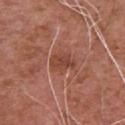Notes:
* workup: catalogued during a skin exam; not biopsied
* image source: ~15 mm tile from a whole-body skin photo
* illumination: white-light
* automated metrics: a mean CIELAB color near L≈44 a*≈25 b*≈29, a lesion–skin lightness drop of about 8, and a normalized border contrast of about 6.5; border irregularity of about 2.5 on a 0–10 scale; a nevus-likeness score of about 5/100 and a detector confidence of about 100 out of 100 that the crop contains a lesion
* lesion size: ≈3 mm
* patient: male, aged approximately 75
* body site: the chest